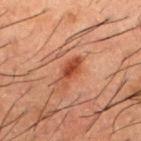Image and clinical context: A close-up tile cropped from a whole-body skin photograph, about 15 mm across. The lesion is on the upper back. A male subject, aged approximately 50. Imaged with cross-polarized lighting.